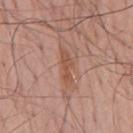Q: Was a biopsy performed?
A: catalogued during a skin exam; not biopsied
Q: Who is the patient?
A: male, aged approximately 65
Q: How was this image acquired?
A: ~15 mm crop, total-body skin-cancer survey
Q: What lighting was used for the tile?
A: white-light
Q: Lesion location?
A: the mid back
Q: What is the lesion's diameter?
A: about 4.5 mm